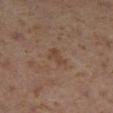Recorded during total-body skin imaging; not selected for excision or biopsy. This is a cross-polarized tile. A female patient in their 40s. Located on the right lower leg. A region of skin cropped from a whole-body photographic capture, roughly 15 mm wide. The lesion-visualizer software estimated a footprint of about 4 mm², an eccentricity of roughly 0.75, and a shape-asymmetry score of about 0.3 (0 = symmetric). And it measured a border-irregularity index near 3.5/10, a color-variation rating of about 2/10, and radial color variation of about 0.5.